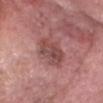notes = catalogued during a skin exam; not biopsied
size = ~5 mm (longest diameter)
site = the head or neck
acquisition = ~15 mm crop, total-body skin-cancer survey
tile lighting = white-light illumination
subject = male, about 75 years old